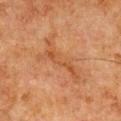Recorded during total-body skin imaging; not selected for excision or biopsy.
Imaged with cross-polarized lighting.
The subject is a male aged around 80.
The lesion's longest dimension is about 7.5 mm.
Automated tile analysis of the lesion measured an area of roughly 14 mm². The software also gave a lesion color around L≈42 a*≈20 b*≈32 in CIELAB, about 5 CIELAB-L* units darker than the surrounding skin, and a normalized border contrast of about 5. The software also gave a nevus-likeness score of about 0/100.
The lesion is located on the front of the torso.
This image is a 15 mm lesion crop taken from a total-body photograph.